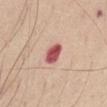Q: Was this lesion biopsied?
A: total-body-photography surveillance lesion; no biopsy
Q: Who is the patient?
A: male, aged approximately 65
Q: What kind of image is this?
A: 15 mm crop, total-body photography
Q: Where on the body is the lesion?
A: the left thigh
Q: Lesion size?
A: ~2.5 mm (longest diameter)
Q: What did automated image analysis measure?
A: a shape eccentricity near 0.6 and a shape-asymmetry score of about 0.2 (0 = symmetric); an automated nevus-likeness rating near 0 out of 100 and lesion-presence confidence of about 100/100